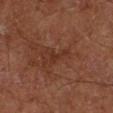<record>
<biopsy_status>not biopsied; imaged during a skin examination</biopsy_status>
<lighting>cross-polarized</lighting>
<image>
  <source>total-body photography crop</source>
  <field_of_view_mm>15</field_of_view_mm>
</image>
<patient>
  <sex>male</sex>
  <age_approx>65</age_approx>
</patient>
<site>left lower leg</site>
<lesion_size>
  <long_diameter_mm_approx>3.0</long_diameter_mm_approx>
</lesion_size>
</record>